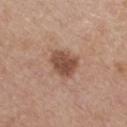Recorded during total-body skin imaging; not selected for excision or biopsy.
A 15 mm crop from a total-body photograph taken for skin-cancer surveillance.
The recorded lesion diameter is about 3.5 mm.
The lesion is on the chest.
A female subject about 45 years old.
Captured under white-light illumination.